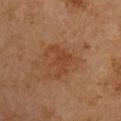workup=catalogued during a skin exam; not biopsied
size=~4 mm (longest diameter)
location=the left arm
subject=male, about 65 years old
imaging modality=15 mm crop, total-body photography
illumination=cross-polarized illumination